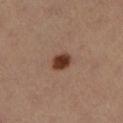Clinical impression:
No biopsy was performed on this lesion — it was imaged during a full skin examination and was not determined to be concerning.
Image and clinical context:
Measured at roughly 2.5 mm in maximum diameter. A 15 mm crop from a total-body photograph taken for skin-cancer surveillance. The lesion is on the leg. Imaged with cross-polarized lighting. The patient is a female aged around 35.